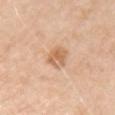{"biopsy_status": "not biopsied; imaged during a skin examination", "image": {"source": "total-body photography crop", "field_of_view_mm": 15}, "patient": {"sex": "male", "age_approx": 70}, "lighting": "white-light", "automated_metrics": {"area_mm2_approx": 5.5, "eccentricity": 0.65, "shape_asymmetry": 0.2, "vs_skin_darker_L": 10.0, "vs_skin_contrast_norm": 6.5}, "site": "right upper arm", "lesion_size": {"long_diameter_mm_approx": 2.5}}